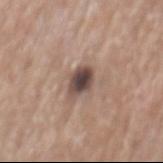This lesion was catalogued during total-body skin photography and was not selected for biopsy. The subject is a male aged around 70. The lesion is located on the mid back. A 15 mm close-up extracted from a 3D total-body photography capture.